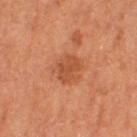| key | value |
|---|---|
| follow-up | imaged on a skin check; not biopsied |
| subject | female, approximately 60 years of age |
| image source | 15 mm crop, total-body photography |
| image-analysis metrics | an area of roughly 7 mm², a shape eccentricity near 0.55, and two-axis asymmetry of about 0.25 |
| body site | the left thigh |
| diameter | about 3.5 mm |
| tile lighting | cross-polarized illumination |